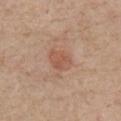{"site": "chest", "lesion_size": {"long_diameter_mm_approx": 3.0}, "patient": {"sex": "male", "age_approx": 75}, "image": {"source": "total-body photography crop", "field_of_view_mm": 15}}